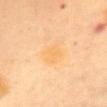biopsy_status: not biopsied; imaged during a skin examination
image:
  source: total-body photography crop
  field_of_view_mm: 15
patient:
  sex: female
  age_approx: 60
site: chest
lesion_size:
  long_diameter_mm_approx: 3.5
lighting: cross-polarized